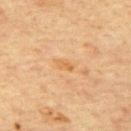<tbp_lesion>
  <biopsy_status>not biopsied; imaged during a skin examination</biopsy_status>
  <lighting>cross-polarized</lighting>
  <site>upper back</site>
  <automated_metrics>
    <vs_skin_darker_L>6.0</vs_skin_darker_L>
    <vs_skin_contrast_norm>5.5</vs_skin_contrast_norm>
    <nevus_likeness_0_100>0</nevus_likeness_0_100>
    <lesion_detection_confidence_0_100>100</lesion_detection_confidence_0_100>
  </automated_metrics>
  <patient>
    <sex>male</sex>
    <age_approx>65</age_approx>
  </patient>
  <image>
    <source>total-body photography crop</source>
    <field_of_view_mm>15</field_of_view_mm>
  </image>
  <lesion_size>
    <long_diameter_mm_approx>2.5</long_diameter_mm_approx>
  </lesion_size>
</tbp_lesion>